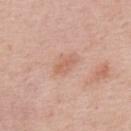Clinical impression:
The lesion was tiled from a total-body skin photograph and was not biopsied.
Acquisition and patient details:
Approximately 3 mm at its widest. The lesion is on the upper back. The total-body-photography lesion software estimated an area of roughly 3 mm², an eccentricity of roughly 0.9, and two-axis asymmetry of about 0.35. It also reported roughly 8 lightness units darker than nearby skin and a normalized border contrast of about 5.5. And it measured a border-irregularity rating of about 3.5/10, internal color variation of about 1 on a 0–10 scale, and radial color variation of about 0. It also reported an automated nevus-likeness rating near 5 out of 100 and a lesion-detection confidence of about 100/100. This is a white-light tile. A 15 mm close-up extracted from a 3D total-body photography capture. A male subject, approximately 75 years of age.